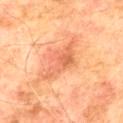Captured during whole-body skin photography for melanoma surveillance; the lesion was not biopsied. The lesion is located on the mid back. A male subject aged approximately 75. Approximately 5.5 mm at its widest. Captured under cross-polarized illumination. A region of skin cropped from a whole-body photographic capture, roughly 15 mm wide.